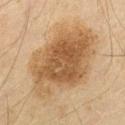Imaged during a routine full-body skin examination; the lesion was not biopsied and no histopathology is available.
A male subject, about 60 years old.
Approximately 10.5 mm at its widest.
A lesion tile, about 15 mm wide, cut from a 3D total-body photograph.
Imaged with cross-polarized lighting.
The total-body-photography lesion software estimated a lesion area of about 55 mm² and a shape eccentricity near 0.7. The software also gave an average lesion color of about L≈47 a*≈14 b*≈31 (CIELAB), a lesion–skin lightness drop of about 10, and a normalized border contrast of about 8. The analysis additionally found a border-irregularity rating of about 2.5/10 and a within-lesion color-variation index near 5.5/10.
The lesion is located on the left thigh.